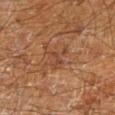biopsy status: no biopsy performed (imaged during a skin exam); tile lighting: cross-polarized; patient: male, approximately 60 years of age; image: ~15 mm crop, total-body skin-cancer survey; site: the left lower leg.